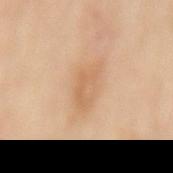biopsy status: imaged on a skin check; not biopsied | patient: female, in their 60s | imaging modality: ~15 mm crop, total-body skin-cancer survey | lighting: cross-polarized illumination | lesion diameter: ~4.5 mm (longest diameter) | site: the mid back | automated metrics: a lesion area of about 9 mm², an outline eccentricity of about 0.85 (0 = round, 1 = elongated), and a shape-asymmetry score of about 0.25 (0 = symmetric); a border-irregularity rating of about 3/10, a color-variation rating of about 2.5/10, and peripheral color asymmetry of about 1.